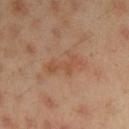Recorded during total-body skin imaging; not selected for excision or biopsy. Cropped from a total-body skin-imaging series; the visible field is about 15 mm. The subject is a male aged approximately 45. Automated tile analysis of the lesion measured a lesion color around L≈50 a*≈20 b*≈32 in CIELAB, a lesion–skin lightness drop of about 6, and a normalized lesion–skin contrast near 5. The analysis additionally found a nevus-likeness score of about 0/100 and lesion-presence confidence of about 100/100. Located on the right upper arm. The lesion's longest dimension is about 5 mm. Captured under cross-polarized illumination.